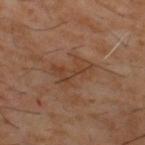Impression:
The lesion was photographed on a routine skin check and not biopsied; there is no pathology result.
Image and clinical context:
This is a cross-polarized tile. A male patient, roughly 60 years of age. The lesion is located on the upper back. A close-up tile cropped from a whole-body skin photograph, about 15 mm across.